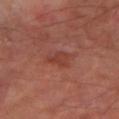Part of a total-body skin-imaging series; this lesion was reviewed on a skin check and was not flagged for biopsy. A 15 mm close-up tile from a total-body photography series done for melanoma screening. The lesion is on the left thigh. Automated tile analysis of the lesion measured roughly 6 lightness units darker than nearby skin. It also reported a within-lesion color-variation index near 2.5/10 and a peripheral color-asymmetry measure near 1. The software also gave an automated nevus-likeness rating near 0 out of 100 and lesion-presence confidence of about 100/100. A male patient, approximately 70 years of age. The tile uses cross-polarized illumination.